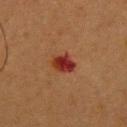The lesion was photographed on a routine skin check and not biopsied; there is no pathology result. A female patient, aged 38–42. This image is a 15 mm lesion crop taken from a total-body photograph. From the head or neck.